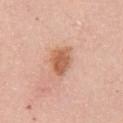Imaged during a routine full-body skin examination; the lesion was not biopsied and no histopathology is available.
The lesion is located on the chest.
A close-up tile cropped from a whole-body skin photograph, about 15 mm across.
Automated tile analysis of the lesion measured a lesion area of about 8 mm², an outline eccentricity of about 0.7 (0 = round, 1 = elongated), and two-axis asymmetry of about 0.25.
A female patient aged 63 to 67.
The recorded lesion diameter is about 4 mm.